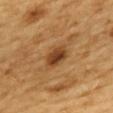– notes · imaged on a skin check; not biopsied
– automated metrics · a shape eccentricity near 0.7 and two-axis asymmetry of about 0.15; an automated nevus-likeness rating near 85 out of 100 and a detector confidence of about 100 out of 100 that the crop contains a lesion
– imaging modality · ~15 mm tile from a whole-body skin photo
– lesion size · ~3 mm (longest diameter)
– subject · female, approximately 55 years of age
– illumination · cross-polarized
– body site · the upper back